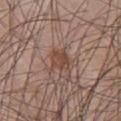No biopsy was performed on this lesion — it was imaged during a full skin examination and was not determined to be concerning.
The total-body-photography lesion software estimated an automated nevus-likeness rating near 75 out of 100 and a lesion-detection confidence of about 100/100.
This is a white-light tile.
A roughly 15 mm field-of-view crop from a total-body skin photograph.
The patient is a male about 60 years old.
Longest diameter approximately 2.5 mm.
The lesion is located on the chest.